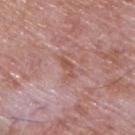The lesion was tiled from a total-body skin photograph and was not biopsied. The lesion is on the upper back. The lesion's longest dimension is about 3 mm. A roughly 15 mm field-of-view crop from a total-body skin photograph. A male subject aged approximately 65. An algorithmic analysis of the crop reported an automated nevus-likeness rating near 0 out of 100. This is a white-light tile.